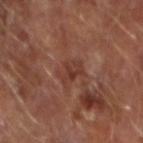follow-up = total-body-photography surveillance lesion; no biopsy
subject = male, roughly 65 years of age
imaging modality = ~15 mm crop, total-body skin-cancer survey
location = the right forearm
size = ≈3.5 mm
tile lighting = cross-polarized